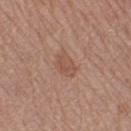Clinical impression:
The lesion was tiled from a total-body skin photograph and was not biopsied.